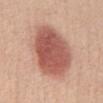This lesion was catalogued during total-body skin photography and was not selected for biopsy. Automated tile analysis of the lesion measured a mean CIELAB color near L≈55 a*≈26 b*≈28, roughly 15 lightness units darker than nearby skin, and a normalized border contrast of about 9.5. The software also gave a nevus-likeness score of about 100/100 and a detector confidence of about 100 out of 100 that the crop contains a lesion. The patient is a female about 50 years old. The lesion is located on the abdomen. Cropped from a whole-body photographic skin survey; the tile spans about 15 mm. This is a white-light tile. About 7.5 mm across.